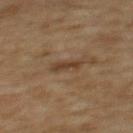{
  "image": {
    "source": "total-body photography crop",
    "field_of_view_mm": 15
  },
  "patient": {
    "sex": "female",
    "age_approx": 60
  },
  "lighting": "cross-polarized",
  "lesion_size": {
    "long_diameter_mm_approx": 3.0
  },
  "site": "back"
}